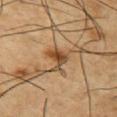{"biopsy_status": "not biopsied; imaged during a skin examination", "lighting": "cross-polarized", "image": {"source": "total-body photography crop", "field_of_view_mm": 15}, "patient": {"sex": "male", "age_approx": 55}, "site": "front of the torso", "automated_metrics": {"nevus_likeness_0_100": 95, "lesion_detection_confidence_0_100": 100}}